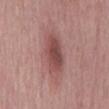image source: 15 mm crop, total-body photography | site: the mid back | size: about 6 mm | subject: male, aged approximately 60 | lighting: white-light illumination.